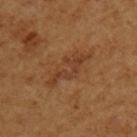The tile uses cross-polarized illumination. From the left upper arm. A close-up tile cropped from a whole-body skin photograph, about 15 mm across. The subject is a female aged 53–57. Approximately 6 mm at its widest. Automated tile analysis of the lesion measured a detector confidence of about 95 out of 100 that the crop contains a lesion.